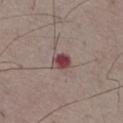From the right thigh.
The lesion-visualizer software estimated an area of roughly 4 mm², an eccentricity of roughly 0.7, and a shape-asymmetry score of about 0.2 (0 = symmetric). It also reported a mean CIELAB color near L≈43 a*≈24 b*≈17 and a lesion–skin lightness drop of about 14. It also reported a border-irregularity rating of about 2/10, a color-variation rating of about 4/10, and peripheral color asymmetry of about 1.
A male subject, in their mid-70s.
The lesion's longest dimension is about 3 mm.
A 15 mm close-up extracted from a 3D total-body photography capture.
This is a white-light tile.